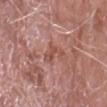This lesion was catalogued during total-body skin photography and was not selected for biopsy.
On the right forearm.
An algorithmic analysis of the crop reported a lesion color around L≈51 a*≈25 b*≈26 in CIELAB, about 8 CIELAB-L* units darker than the surrounding skin, and a lesion-to-skin contrast of about 6 (normalized; higher = more distinct). The software also gave a border-irregularity rating of about 8.5/10, a within-lesion color-variation index near 0/10, and peripheral color asymmetry of about 0. The analysis additionally found an automated nevus-likeness rating near 0 out of 100 and a lesion-detection confidence of about 95/100.
Cropped from a total-body skin-imaging series; the visible field is about 15 mm.
The lesion's longest dimension is about 4 mm.
A male patient, in their mid-70s.